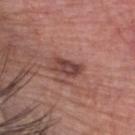The lesion was tiled from a total-body skin photograph and was not biopsied. This is a white-light tile. The total-body-photography lesion software estimated an area of roughly 5.5 mm². It also reported a lesion–skin lightness drop of about 11 and a normalized lesion–skin contrast near 9. It also reported a color-variation rating of about 4/10 and a peripheral color-asymmetry measure near 1.5. It also reported a classifier nevus-likeness of about 20/100 and a lesion-detection confidence of about 100/100. The lesion is on the head or neck. About 3 mm across. A close-up tile cropped from a whole-body skin photograph, about 15 mm across. A male patient aged 63 to 67.